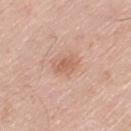* notes: no biopsy performed (imaged during a skin exam)
* lesion size: ≈3 mm
* image-analysis metrics: an area of roughly 5 mm², an eccentricity of roughly 0.65, and a shape-asymmetry score of about 0.25 (0 = symmetric); a lesion–skin lightness drop of about 8 and a lesion-to-skin contrast of about 6 (normalized; higher = more distinct); an automated nevus-likeness rating near 10 out of 100
* image: ~15 mm crop, total-body skin-cancer survey
* site: the leg
* patient: male, approximately 80 years of age